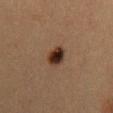Background: A female patient aged around 40. The recorded lesion diameter is about 3.5 mm. Captured under cross-polarized illumination. Automated tile analysis of the lesion measured a classifier nevus-likeness of about 100/100 and lesion-presence confidence of about 100/100. Cropped from a whole-body photographic skin survey; the tile spans about 15 mm. The lesion is on the mid back.